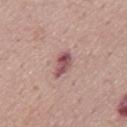Captured during whole-body skin photography for melanoma surveillance; the lesion was not biopsied.